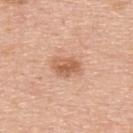notes: total-body-photography surveillance lesion; no biopsy
TBP lesion metrics: a lesion area of about 6.5 mm², an eccentricity of roughly 0.75, and a symmetry-axis asymmetry near 0.2; an average lesion color of about L≈60 a*≈23 b*≈34 (CIELAB)
illumination: white-light
image: ~15 mm crop, total-body skin-cancer survey
patient: male, aged approximately 50
anatomic site: the upper back